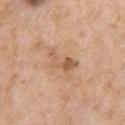Impression: Captured during whole-body skin photography for melanoma surveillance; the lesion was not biopsied. Clinical summary: Located on the right upper arm. Approximately 4 mm at its widest. The tile uses white-light illumination. The subject is a female aged around 75. The total-body-photography lesion software estimated a footprint of about 5.5 mm² and a shape eccentricity near 0.85. And it measured a lesion color around L≈58 a*≈21 b*≈33 in CIELAB, about 10 CIELAB-L* units darker than the surrounding skin, and a normalized border contrast of about 6.5. A roughly 15 mm field-of-view crop from a total-body skin photograph.